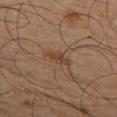No biopsy was performed on this lesion — it was imaged during a full skin examination and was not determined to be concerning. On the left thigh. The tile uses cross-polarized illumination. Cropped from a whole-body photographic skin survey; the tile spans about 15 mm. A male subject about 65 years old. The recorded lesion diameter is about 3 mm.